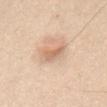Recorded during total-body skin imaging; not selected for excision or biopsy.
On the chest.
Imaged with white-light lighting.
The lesion-visualizer software estimated roughly 10 lightness units darker than nearby skin and a normalized lesion–skin contrast near 6. And it measured a border-irregularity index near 4/10, internal color variation of about 2 on a 0–10 scale, and radial color variation of about 0.5.
Cropped from a total-body skin-imaging series; the visible field is about 15 mm.
Approximately 3 mm at its widest.
The patient is a male aged approximately 45.